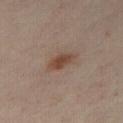Q: Is there a histopathology result?
A: total-body-photography surveillance lesion; no biopsy
Q: How was the tile lit?
A: cross-polarized
Q: What did automated image analysis measure?
A: a footprint of about 4.5 mm²
Q: How large is the lesion?
A: ~3.5 mm (longest diameter)
Q: Where on the body is the lesion?
A: the front of the torso
Q: Who is the patient?
A: female, aged 33 to 37
Q: How was this image acquired?
A: 15 mm crop, total-body photography